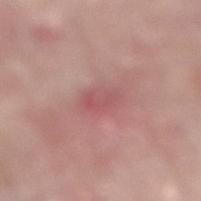The lesion was photographed on a routine skin check and not biopsied; there is no pathology result. Longest diameter approximately 3.5 mm. A female subject, roughly 65 years of age. This is a white-light tile. From the left leg. A 15 mm crop from a total-body photograph taken for skin-cancer surveillance.